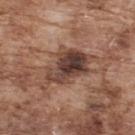Notes:
* biopsy status · catalogued during a skin exam; not biopsied
* patient · male, aged around 75
* lesion diameter · ~7 mm (longest diameter)
* location · the upper back
* image · 15 mm crop, total-body photography
* illumination · white-light illumination